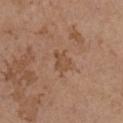| field | value |
|---|---|
| follow-up | no biopsy performed (imaged during a skin exam) |
| subject | female, about 65 years old |
| body site | the chest |
| size | ≈2.5 mm |
| imaging modality | ~15 mm crop, total-body skin-cancer survey |